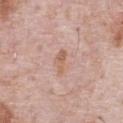<lesion>
  <biopsy_status>not biopsied; imaged during a skin examination</biopsy_status>
  <lighting>white-light</lighting>
  <site>chest</site>
  <image>
    <source>total-body photography crop</source>
    <field_of_view_mm>15</field_of_view_mm>
  </image>
  <automated_metrics>
    <border_irregularity_0_10>4.5</border_irregularity_0_10>
    <color_variation_0_10>2.0</color_variation_0_10>
    <peripheral_color_asymmetry>0.5</peripheral_color_asymmetry>
  </automated_metrics>
  <patient>
    <sex>male</sex>
    <age_approx>70</age_approx>
  </patient>
</lesion>